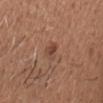biopsy status: total-body-photography surveillance lesion; no biopsy | body site: the head or neck | subject: male, aged around 50 | acquisition: total-body-photography crop, ~15 mm field of view.